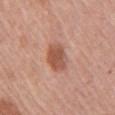Q: Illumination type?
A: white-light illumination
Q: Who is the patient?
A: female, roughly 65 years of age
Q: How was this image acquired?
A: 15 mm crop, total-body photography
Q: What is the anatomic site?
A: the right upper arm
Q: How large is the lesion?
A: about 3.5 mm